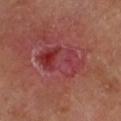This lesion was catalogued during total-body skin photography and was not selected for biopsy.
A 15 mm close-up tile from a total-body photography series done for melanoma screening.
About 6 mm across.
Located on the leg.
A male patient, roughly 65 years of age.